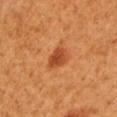{
  "biopsy_status": "not biopsied; imaged during a skin examination",
  "automated_metrics": {
    "border_irregularity_0_10": 2.0,
    "color_variation_0_10": 2.5
  },
  "site": "left upper arm",
  "image": {
    "source": "total-body photography crop",
    "field_of_view_mm": 15
  },
  "patient": {
    "sex": "male",
    "age_approx": 50
  },
  "lighting": "cross-polarized",
  "lesion_size": {
    "long_diameter_mm_approx": 3.0
  }
}